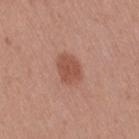Notes:
– notes — no biopsy performed (imaged during a skin exam)
– anatomic site — the right thigh
– tile lighting — white-light illumination
– TBP lesion metrics — a mean CIELAB color near L≈52 a*≈24 b*≈29 and a lesion-to-skin contrast of about 7 (normalized; higher = more distinct)
– size — ≈3.5 mm
– subject — female, approximately 40 years of age
– image source — total-body-photography crop, ~15 mm field of view